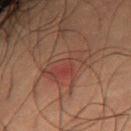– biopsy status · imaged on a skin check; not biopsied
– diameter · ~6 mm (longest diameter)
– anatomic site · the lower back
– subject · female, aged around 80
– acquisition · total-body-photography crop, ~15 mm field of view
– automated lesion analysis · a lesion color around L≈30 a*≈18 b*≈20 in CIELAB and roughly 4 lightness units darker than nearby skin; a within-lesion color-variation index near 3.5/10 and peripheral color asymmetry of about 1
– tile lighting · cross-polarized